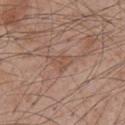No biopsy was performed on this lesion — it was imaged during a full skin examination and was not determined to be concerning.
The lesion is on the abdomen.
Imaged with white-light lighting.
A male patient, aged around 60.
Longest diameter approximately 2.5 mm.
A 15 mm close-up tile from a total-body photography series done for melanoma screening.
An algorithmic analysis of the crop reported a footprint of about 4 mm², an eccentricity of roughly 0.65, and two-axis asymmetry of about 0.45. And it measured a normalized lesion–skin contrast near 5. And it measured an automated nevus-likeness rating near 0 out of 100.